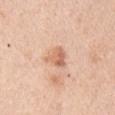A region of skin cropped from a whole-body photographic capture, roughly 15 mm wide.
On the right upper arm.
Approximately 3 mm at its widest.
A male patient, in their 50s.
Automated image analysis of the tile measured a lesion area of about 5 mm², a shape eccentricity near 0.4, and a shape-asymmetry score of about 0.4 (0 = symmetric). The software also gave a color-variation rating of about 4/10 and radial color variation of about 1.5.
Captured under white-light illumination.